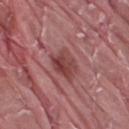About 3.5 mm across. The lesion is located on the right thigh. The patient is a male aged 38 to 42. Automated image analysis of the tile measured a footprint of about 8.5 mm², a shape eccentricity near 0.4, and two-axis asymmetry of about 0.25. The analysis additionally found an average lesion color of about L≈43 a*≈27 b*≈21 (CIELAB), roughly 10 lightness units darker than nearby skin, and a normalized border contrast of about 8. The software also gave border irregularity of about 3 on a 0–10 scale, internal color variation of about 6 on a 0–10 scale, and peripheral color asymmetry of about 2. A region of skin cropped from a whole-body photographic capture, roughly 15 mm wide.